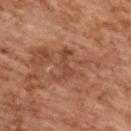{
  "biopsy_status": "not biopsied; imaged during a skin examination",
  "site": "upper back",
  "patient": {
    "sex": "female",
    "age_approx": 60
  },
  "image": {
    "source": "total-body photography crop",
    "field_of_view_mm": 15
  }
}